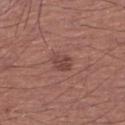Case summary:
• follow-up: no biopsy performed (imaged during a skin exam)
• patient: male, roughly 65 years of age
• anatomic site: the right lower leg
• acquisition: ~15 mm crop, total-body skin-cancer survey
• tile lighting: white-light illumination